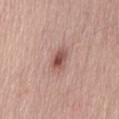This lesion was catalogued during total-body skin photography and was not selected for biopsy.
The lesion is located on the mid back.
An algorithmic analysis of the crop reported a mean CIELAB color near L≈52 a*≈23 b*≈24 and roughly 12 lightness units darker than nearby skin. The analysis additionally found border irregularity of about 2 on a 0–10 scale, a within-lesion color-variation index near 5.5/10, and peripheral color asymmetry of about 2. It also reported a detector confidence of about 100 out of 100 that the crop contains a lesion.
This is a white-light tile.
A lesion tile, about 15 mm wide, cut from a 3D total-body photograph.
A male patient roughly 65 years of age.
About 3 mm across.